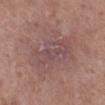{"biopsy_status": "not biopsied; imaged during a skin examination", "site": "right lower leg", "lesion_size": {"long_diameter_mm_approx": 6.0}, "automated_metrics": {"cielab_L": 48, "cielab_a": 20, "cielab_b": 17, "vs_skin_darker_L": 6.0, "vs_skin_contrast_norm": 5.5, "border_irregularity_0_10": 4.5, "color_variation_0_10": 4.0, "peripheral_color_asymmetry": 1.5, "lesion_detection_confidence_0_100": 85}, "image": {"source": "total-body photography crop", "field_of_view_mm": 15}, "patient": {"sex": "female", "age_approx": 50}}